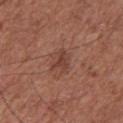Clinical impression: The lesion was photographed on a routine skin check and not biopsied; there is no pathology result. Context: A male patient roughly 75 years of age. A region of skin cropped from a whole-body photographic capture, roughly 15 mm wide. The lesion is on the chest. The tile uses white-light illumination.